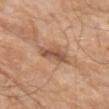notes: imaged on a skin check; not biopsied
lesion diameter: ≈4.5 mm
image-analysis metrics: an area of roughly 7 mm², an outline eccentricity of about 0.9 (0 = round, 1 = elongated), and a shape-asymmetry score of about 0.35 (0 = symmetric); a lesion color around L≈53 a*≈20 b*≈31 in CIELAB and a normalized lesion–skin contrast near 7.5; border irregularity of about 4 on a 0–10 scale, internal color variation of about 4.5 on a 0–10 scale, and a peripheral color-asymmetry measure near 1.5; a nevus-likeness score of about 5/100
image source: 15 mm crop, total-body photography
illumination: white-light illumination
location: the left upper arm
patient: male, in their 80s